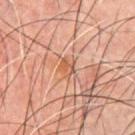  biopsy_status: not biopsied; imaged during a skin examination
  patient:
    sex: male
    age_approx: 45
  image:
    source: total-body photography crop
    field_of_view_mm: 15
  site: chest
  lighting: cross-polarized